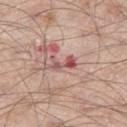biopsy status: no biopsy performed (imaged during a skin exam) | location: the left thigh | lesion size: ~3 mm (longest diameter) | tile lighting: white-light | image: total-body-photography crop, ~15 mm field of view | subject: male, about 60 years old.